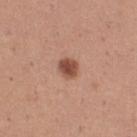Notes:
• workup · imaged on a skin check; not biopsied
• patient · female, aged 28–32
• size · ≈2.5 mm
• image source · total-body-photography crop, ~15 mm field of view
• anatomic site · the right thigh
• illumination · white-light illumination
• automated metrics · a lesion color around L≈50 a*≈23 b*≈29 in CIELAB, about 14 CIELAB-L* units darker than the surrounding skin, and a lesion-to-skin contrast of about 9.5 (normalized; higher = more distinct); an automated nevus-likeness rating near 95 out of 100 and a lesion-detection confidence of about 100/100